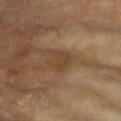Q: Is there a histopathology result?
A: imaged on a skin check; not biopsied
Q: Lesion location?
A: the left upper arm
Q: Automated lesion metrics?
A: a border-irregularity rating of about 4/10, internal color variation of about 2 on a 0–10 scale, and a peripheral color-asymmetry measure near 0.5
Q: What is the imaging modality?
A: total-body-photography crop, ~15 mm field of view
Q: Patient demographics?
A: female, aged approximately 75
Q: Illumination type?
A: cross-polarized
Q: How large is the lesion?
A: ~3.5 mm (longest diameter)